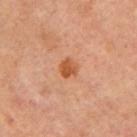{"biopsy_status": "not biopsied; imaged during a skin examination", "lesion_size": {"long_diameter_mm_approx": 2.5}, "image": {"source": "total-body photography crop", "field_of_view_mm": 15}, "site": "arm", "automated_metrics": {"cielab_L": 57, "cielab_a": 29, "cielab_b": 41, "vs_skin_darker_L": 11.0, "vs_skin_contrast_norm": 8.5, "border_irregularity_0_10": 1.5, "color_variation_0_10": 3.0, "peripheral_color_asymmetry": 1.0, "nevus_likeness_0_100": 70, "lesion_detection_confidence_0_100": 100}, "patient": {"sex": "female", "age_approx": 60}}